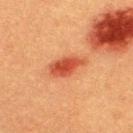Captured under cross-polarized illumination. This image is a 15 mm lesion crop taken from a total-body photograph. The lesion is on the back. The subject is a male aged 38 to 42.